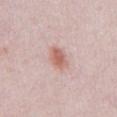workup=imaged on a skin check; not biopsied | lesion size=about 3.5 mm | patient=male, aged approximately 25 | acquisition=total-body-photography crop, ~15 mm field of view | illumination=white-light | location=the chest.